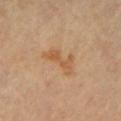follow-up: total-body-photography surveillance lesion; no biopsy
lesion size: ≈4 mm
anatomic site: the arm
patient: male, roughly 65 years of age
image: 15 mm crop, total-body photography
tile lighting: cross-polarized